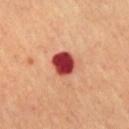The lesion was photographed on a routine skin check and not biopsied; there is no pathology result.
A lesion tile, about 15 mm wide, cut from a 3D total-body photograph.
The tile uses cross-polarized illumination.
Longest diameter approximately 3 mm.
The lesion is on the front of the torso.
The patient is a male approximately 70 years of age.